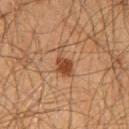| feature | finding |
|---|---|
| biopsy status | catalogued during a skin exam; not biopsied |
| lesion size | ~3.5 mm (longest diameter) |
| patient | male, aged approximately 60 |
| acquisition | 15 mm crop, total-body photography |
| site | the chest |